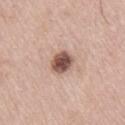Clinical impression: Captured during whole-body skin photography for melanoma surveillance; the lesion was not biopsied. Image and clinical context: Located on the right thigh. This image is a 15 mm lesion crop taken from a total-body photograph. A male subject about 55 years old. Approximately 3 mm at its widest. Automated tile analysis of the lesion measured an eccentricity of roughly 0.35. And it measured a border-irregularity rating of about 2/10, internal color variation of about 4 on a 0–10 scale, and peripheral color asymmetry of about 1. Imaged with white-light lighting.